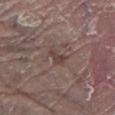Part of a total-body skin-imaging series; this lesion was reviewed on a skin check and was not flagged for biopsy. On the left lower leg. A male patient, approximately 80 years of age. The tile uses white-light illumination. A region of skin cropped from a whole-body photographic capture, roughly 15 mm wide. The recorded lesion diameter is about 2.5 mm.